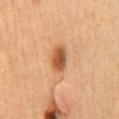Case summary:
• follow-up — total-body-photography surveillance lesion; no biopsy